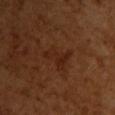Q: Was this lesion biopsied?
A: no biopsy performed (imaged during a skin exam)
Q: How large is the lesion?
A: ~4.5 mm (longest diameter)
Q: What are the patient's age and sex?
A: female, aged approximately 55
Q: What lighting was used for the tile?
A: cross-polarized
Q: Automated lesion metrics?
A: a lesion color around L≈25 a*≈22 b*≈28 in CIELAB, roughly 5 lightness units darker than nearby skin, and a normalized lesion–skin contrast near 5.5
Q: What is the anatomic site?
A: the upper back
Q: How was this image acquired?
A: ~15 mm crop, total-body skin-cancer survey